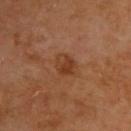Clinical impression:
Imaged during a routine full-body skin examination; the lesion was not biopsied and no histopathology is available.
Background:
The patient is a male in their mid-60s. A 15 mm close-up extracted from a 3D total-body photography capture. Located on the upper back.